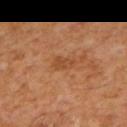The lesion was photographed on a routine skin check and not biopsied; there is no pathology result.
A region of skin cropped from a whole-body photographic capture, roughly 15 mm wide.
The patient is a male in their mid-60s.
Located on the mid back.